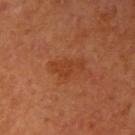subject = female, aged approximately 65
lesion size = about 4 mm
tile lighting = cross-polarized
automated metrics = a border-irregularity index near 4/10, a within-lesion color-variation index near 1.5/10, and a peripheral color-asymmetry measure near 0.5; a classifier nevus-likeness of about 5/100
body site = the right upper arm
image source = 15 mm crop, total-body photography